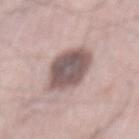Case summary:
- workup · no biopsy performed (imaged during a skin exam)
- image source · total-body-photography crop, ~15 mm field of view
- patient · male, approximately 65 years of age
- diameter · ~6 mm (longest diameter)
- location · the mid back